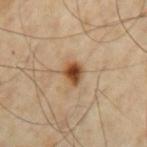Captured during whole-body skin photography for melanoma surveillance; the lesion was not biopsied.
From the arm.
The subject is a male approximately 65 years of age.
This image is a 15 mm lesion crop taken from a total-body photograph.
About 2.5 mm across.
The lesion-visualizer software estimated a border-irregularity rating of about 2/10, internal color variation of about 4.5 on a 0–10 scale, and a peripheral color-asymmetry measure near 1.5. And it measured a nevus-likeness score of about 100/100 and a lesion-detection confidence of about 100/100.